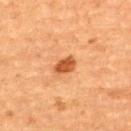Q: Is there a histopathology result?
A: no biopsy performed (imaged during a skin exam)
Q: What lighting was used for the tile?
A: cross-polarized
Q: What is the anatomic site?
A: the upper back
Q: How was this image acquired?
A: total-body-photography crop, ~15 mm field of view
Q: How large is the lesion?
A: ≈3 mm
Q: What are the patient's age and sex?
A: female, in their 70s
Q: What did automated image analysis measure?
A: a within-lesion color-variation index near 2.5/10; a classifier nevus-likeness of about 95/100